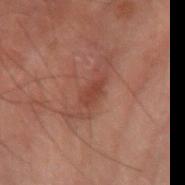Impression: Recorded during total-body skin imaging; not selected for excision or biopsy. Image and clinical context: Automated tile analysis of the lesion measured a lesion area of about 2.5 mm². Imaged with cross-polarized lighting. This image is a 15 mm lesion crop taken from a total-body photograph. A male patient, about 70 years old. The lesion is on the right forearm.